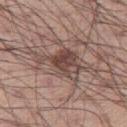  biopsy_status: not biopsied; imaged during a skin examination
  patient:
    sex: male
    age_approx: 55
  lighting: white-light
  automated_metrics:
    cielab_L: 46
    cielab_a: 17
    cielab_b: 21
    vs_skin_darker_L: 11.0
    vs_skin_contrast_norm: 8.5
    border_irregularity_0_10: 6.5
    color_variation_0_10: 6.0
    peripheral_color_asymmetry: 2.0
  lesion_size:
    long_diameter_mm_approx: 6.5
  image:
    source: total-body photography crop
    field_of_view_mm: 15
  site: right thigh